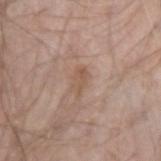follow-up = imaged on a skin check; not biopsied
lighting = white-light illumination
body site = the left forearm
patient = male, in their 50s
imaging modality = ~15 mm tile from a whole-body skin photo
lesion size = ≈3 mm